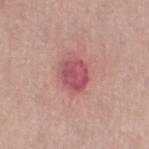The lesion was photographed on a routine skin check and not biopsied; there is no pathology result.
A lesion tile, about 15 mm wide, cut from a 3D total-body photograph.
From the left thigh.
Captured under white-light illumination.
The recorded lesion diameter is about 3.5 mm.
A female subject, aged around 50.